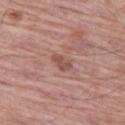• biopsy status — imaged on a skin check; not biopsied
• acquisition — total-body-photography crop, ~15 mm field of view
• anatomic site — the left thigh
• size — ~2.5 mm (longest diameter)
• subject — male, in their mid-70s